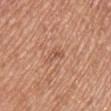Part of a total-body skin-imaging series; this lesion was reviewed on a skin check and was not flagged for biopsy.
A female subject, aged around 65.
On the right upper arm.
The lesion-visualizer software estimated about 8 CIELAB-L* units darker than the surrounding skin and a normalized border contrast of about 5.5. The software also gave a border-irregularity rating of about 5.5/10, a within-lesion color-variation index near 0/10, and radial color variation of about 0. It also reported a nevus-likeness score of about 0/100 and a lesion-detection confidence of about 100/100.
Cropped from a whole-body photographic skin survey; the tile spans about 15 mm.
The lesion's longest dimension is about 2.5 mm.
The tile uses white-light illumination.